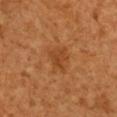notes: total-body-photography surveillance lesion; no biopsy
anatomic site: the right upper arm
diameter: ≈3 mm
lighting: cross-polarized
image source: ~15 mm tile from a whole-body skin photo
subject: male, in their 50s